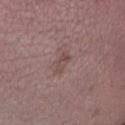  site: lower back
  lesion_size:
    long_diameter_mm_approx: 3.0
  patient:
    sex: male
    age_approx: 45
  automated_metrics:
    cielab_L: 47
    cielab_a: 18
    cielab_b: 20
    vs_skin_contrast_norm: 5.5
    border_irregularity_0_10: 3.0
    peripheral_color_asymmetry: 0.5
    nevus_likeness_0_100: 0
    lesion_detection_confidence_0_100: 100
  image:
    source: total-body photography crop
    field_of_view_mm: 15
  lighting: white-light